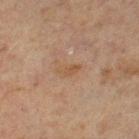Q: Is there a histopathology result?
A: total-body-photography surveillance lesion; no biopsy
Q: What did automated image analysis measure?
A: a lesion area of about 3 mm², a shape eccentricity near 0.9, and two-axis asymmetry of about 0.3; a lesion color around L≈49 a*≈17 b*≈31 in CIELAB, about 6 CIELAB-L* units darker than the surrounding skin, and a lesion-to-skin contrast of about 5.5 (normalized; higher = more distinct)
Q: What kind of image is this?
A: ~15 mm tile from a whole-body skin photo
Q: What is the lesion's diameter?
A: about 2.5 mm
Q: What lighting was used for the tile?
A: cross-polarized
Q: What is the anatomic site?
A: the left lower leg
Q: Patient demographics?
A: male, roughly 60 years of age